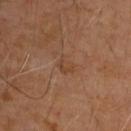  biopsy_status: not biopsied; imaged during a skin examination
  lighting: cross-polarized
  site: upper back
  patient:
    sex: male
    age_approx: 65
  lesion_size:
    long_diameter_mm_approx: 2.5
  image:
    source: total-body photography crop
    field_of_view_mm: 15